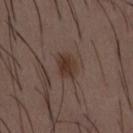Impression: Captured during whole-body skin photography for melanoma surveillance; the lesion was not biopsied. Clinical summary: Cropped from a total-body skin-imaging series; the visible field is about 15 mm. The subject is a male in their 50s. Located on the abdomen.